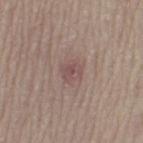workup = no biopsy performed (imaged during a skin exam)
subject = female, aged 58 to 62
automated lesion analysis = a lesion area of about 5 mm² and a symmetry-axis asymmetry near 0.25; a lesion–skin lightness drop of about 7; border irregularity of about 2.5 on a 0–10 scale, a within-lesion color-variation index near 3/10, and radial color variation of about 1; an automated nevus-likeness rating near 0 out of 100 and a detector confidence of about 100 out of 100 that the crop contains a lesion
imaging modality = ~15 mm crop, total-body skin-cancer survey
site = the left thigh
lesion diameter = ~2.5 mm (longest diameter)
illumination = white-light